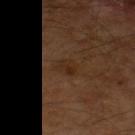This lesion was catalogued during total-body skin photography and was not selected for biopsy.
Captured under cross-polarized illumination.
On the right thigh.
Longest diameter approximately 2.5 mm.
The lesion-visualizer software estimated a footprint of about 3.5 mm², a shape eccentricity near 0.7, and a symmetry-axis asymmetry near 0.3. The analysis additionally found radial color variation of about 1. It also reported an automated nevus-likeness rating near 5 out of 100.
A male patient aged 58–62.
A roughly 15 mm field-of-view crop from a total-body skin photograph.